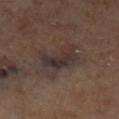diameter: ≈5 mm
location: the left lower leg
automated metrics: an outline eccentricity of about 0.8 (0 = round, 1 = elongated) and a shape-asymmetry score of about 0.35 (0 = symmetric); a border-irregularity rating of about 4.5/10 and radial color variation of about 2
imaging modality: total-body-photography crop, ~15 mm field of view